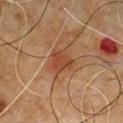This lesion was catalogued during total-body skin photography and was not selected for biopsy.